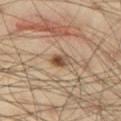From the right thigh.
This image is a 15 mm lesion crop taken from a total-body photograph.
The patient is a male aged around 40.